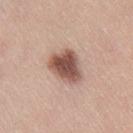follow-up: catalogued during a skin exam; not biopsied | location: the right thigh | patient: female, aged 23 to 27 | acquisition: ~15 mm tile from a whole-body skin photo.